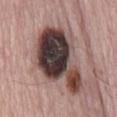The lesion was tiled from a total-body skin photograph and was not biopsied.
A lesion tile, about 15 mm wide, cut from a 3D total-body photograph.
The lesion is located on the mid back.
A male subject, in their mid- to late 60s.
Imaged with white-light lighting.